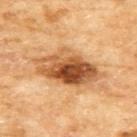This lesion was catalogued during total-body skin photography and was not selected for biopsy.
The patient is a female aged 58 to 62.
The lesion is located on the upper back.
This is a cross-polarized tile.
Approximately 8 mm at its widest.
A lesion tile, about 15 mm wide, cut from a 3D total-body photograph.